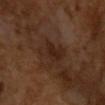<tbp_lesion>
  <biopsy_status>not biopsied; imaged during a skin examination</biopsy_status>
  <image>
    <source>total-body photography crop</source>
    <field_of_view_mm>15</field_of_view_mm>
  </image>
  <patient>
    <sex>male</sex>
    <age_approx>65</age_approx>
  </patient>
</tbp_lesion>